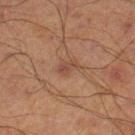* follow-up — no biopsy performed (imaged during a skin exam)
* body site — the left lower leg
* automated metrics — an average lesion color of about L≈37 a*≈18 b*≈23 (CIELAB), a lesion–skin lightness drop of about 6, and a normalized border contrast of about 6
* subject — male, in their 70s
* imaging modality — ~15 mm tile from a whole-body skin photo
* lesion diameter — ≈2.5 mm
* tile lighting — cross-polarized